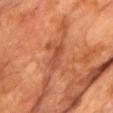  biopsy_status: not biopsied; imaged during a skin examination
  patient:
    sex: male
    age_approx: 75
  lighting: cross-polarized
  automated_metrics:
    area_mm2_approx: 4.0
    eccentricity: 0.95
    shape_asymmetry: 0.35
    vs_skin_contrast_norm: 6.0
    nevus_likeness_0_100: 0
  image:
    source: total-body photography crop
    field_of_view_mm: 15
  site: chest
  lesion_size:
    long_diameter_mm_approx: 3.5